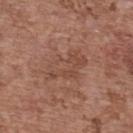{"image": {"source": "total-body photography crop", "field_of_view_mm": 15}, "lesion_size": {"long_diameter_mm_approx": 5.0}, "lighting": "white-light", "patient": {"sex": "female", "age_approx": 65}, "site": "upper back"}